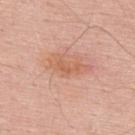{"biopsy_status": "not biopsied; imaged during a skin examination", "automated_metrics": {"area_mm2_approx": 4.0, "shape_asymmetry": 0.35, "cielab_L": 61, "cielab_a": 25, "cielab_b": 34, "vs_skin_darker_L": 7.0, "vs_skin_contrast_norm": 5.5, "lesion_detection_confidence_0_100": 100}, "site": "upper back", "lesion_size": {"long_diameter_mm_approx": 3.0}, "lighting": "white-light", "image": {"source": "total-body photography crop", "field_of_view_mm": 15}, "patient": {"sex": "male", "age_approx": 65}}